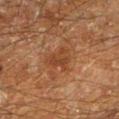biopsy_status: not biopsied; imaged during a skin examination
patient:
  sex: male
  age_approx: 60
site: left lower leg
lighting: cross-polarized
image:
  source: total-body photography crop
  field_of_view_mm: 15
lesion_size:
  long_diameter_mm_approx: 3.5
automated_metrics:
  eccentricity: 0.65
  shape_asymmetry: 0.45
  cielab_L: 32
  cielab_a: 19
  cielab_b: 28
  vs_skin_contrast_norm: 6.0
  border_irregularity_0_10: 4.5
  color_variation_0_10: 3.0
  peripheral_color_asymmetry: 1.0
  nevus_likeness_0_100: 0
  lesion_detection_confidence_0_100: 100